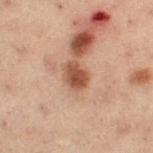{"biopsy_status": "not biopsied; imaged during a skin examination", "patient": {"sex": "female", "age_approx": 55}, "lighting": "cross-polarized", "image": {"source": "total-body photography crop", "field_of_view_mm": 15}, "automated_metrics": {"border_irregularity_0_10": 1.5, "color_variation_0_10": 3.0, "peripheral_color_asymmetry": 1.0}, "site": "leg", "lesion_size": {"long_diameter_mm_approx": 3.0}}